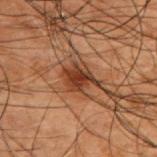Imaged during a routine full-body skin examination; the lesion was not biopsied and no histopathology is available.
A male subject in their 50s.
The tile uses cross-polarized illumination.
The lesion is located on the upper back.
Longest diameter approximately 3.5 mm.
Cropped from a total-body skin-imaging series; the visible field is about 15 mm.